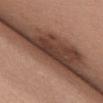Recorded during total-body skin imaging; not selected for excision or biopsy. The lesion-visualizer software estimated border irregularity of about 5.5 on a 0–10 scale, internal color variation of about 1.5 on a 0–10 scale, and peripheral color asymmetry of about 0.5. And it measured a nevus-likeness score of about 0/100 and a detector confidence of about 0 out of 100 that the crop contains a lesion. The subject is a female aged 58 to 62. The recorded lesion diameter is about 6 mm. Cropped from a total-body skin-imaging series; the visible field is about 15 mm. The lesion is on the chest. Captured under white-light illumination.